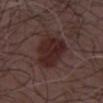patient:
  sex: male
  age_approx: 55
lesion_size:
  long_diameter_mm_approx: 5.0
image:
  source: total-body photography crop
  field_of_view_mm: 15
site: front of the torso
lighting: white-light